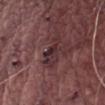workup = no biopsy performed (imaged during a skin exam).